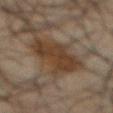workup = catalogued during a skin exam; not biopsied
subject = male, about 65 years old
anatomic site = the abdomen
lighting = cross-polarized illumination
imaging modality = ~15 mm tile from a whole-body skin photo
diameter = about 6.5 mm
automated lesion analysis = a lesion area of about 18 mm² and an eccentricity of roughly 0.8; a border-irregularity index near 4.5/10, internal color variation of about 2.5 on a 0–10 scale, and radial color variation of about 1; an automated nevus-likeness rating near 55 out of 100